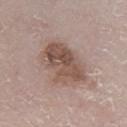This lesion was catalogued during total-body skin photography and was not selected for biopsy.
This is a white-light tile.
The lesion-visualizer software estimated a border-irregularity index near 3/10 and peripheral color asymmetry of about 2. The software also gave a detector confidence of about 100 out of 100 that the crop contains a lesion.
The lesion is located on the right lower leg.
Cropped from a total-body skin-imaging series; the visible field is about 15 mm.
A male patient about 80 years old.